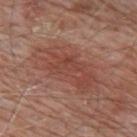The lesion was tiled from a total-body skin photograph and was not biopsied. The subject is a male about 80 years old. The lesion-visualizer software estimated a footprint of about 25 mm² and an eccentricity of roughly 0.9. It also reported a lesion color around L≈46 a*≈24 b*≈27 in CIELAB, a lesion–skin lightness drop of about 7, and a lesion-to-skin contrast of about 6 (normalized; higher = more distinct). The software also gave lesion-presence confidence of about 100/100. Captured under white-light illumination. The lesion's longest dimension is about 8.5 mm. A region of skin cropped from a whole-body photographic capture, roughly 15 mm wide. Located on the mid back.